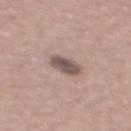Impression: Captured during whole-body skin photography for melanoma surveillance; the lesion was not biopsied. Clinical summary: From the mid back. A male patient, aged 38 to 42. A 15 mm crop from a total-body photograph taken for skin-cancer surveillance.